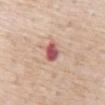notes: total-body-photography surveillance lesion; no biopsy.